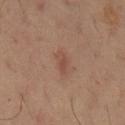Case summary:
* workup · imaged on a skin check; not biopsied
* patient · male, about 60 years old
* acquisition · total-body-photography crop, ~15 mm field of view
* anatomic site · the mid back